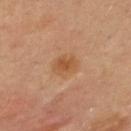Q: Was this lesion biopsied?
A: no biopsy performed (imaged during a skin exam)
Q: How was the tile lit?
A: cross-polarized
Q: What is the lesion's diameter?
A: ≈3 mm
Q: What is the imaging modality?
A: total-body-photography crop, ~15 mm field of view
Q: What are the patient's age and sex?
A: female, aged around 35
Q: Where on the body is the lesion?
A: the back